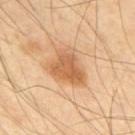biopsy status: imaged on a skin check; not biopsied
patient: male, aged 63 to 67
lighting: cross-polarized
image-analysis metrics: a footprint of about 14 mm² and a shape eccentricity near 0.65; a lesion color around L≈61 a*≈22 b*≈39 in CIELAB, a lesion–skin lightness drop of about 11, and a lesion-to-skin contrast of about 7.5 (normalized; higher = more distinct)
anatomic site: the upper back
image source: ~15 mm crop, total-body skin-cancer survey